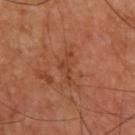  biopsy_status: not biopsied; imaged during a skin examination
  site: upper back
  lighting: cross-polarized
  lesion_size:
    long_diameter_mm_approx: 4.0
  image:
    source: total-body photography crop
    field_of_view_mm: 15
  patient:
    sex: male
    age_approx: 60
  automated_metrics:
    nevus_likeness_0_100: 0
    lesion_detection_confidence_0_100: 100